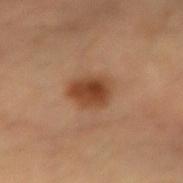  biopsy_status: not biopsied; imaged during a skin examination
  lighting: cross-polarized
  image:
    source: total-body photography crop
    field_of_view_mm: 15
  patient:
    sex: male
    age_approx: 40
  site: left forearm